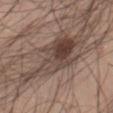Impression:
The lesion was tiled from a total-body skin photograph and was not biopsied.
Image and clinical context:
This image is a 15 mm lesion crop taken from a total-body photograph. The tile uses white-light illumination. Automated image analysis of the tile measured a lesion color around L≈43 a*≈14 b*≈22 in CIELAB and a normalized border contrast of about 9. Longest diameter approximately 9 mm. A male patient in their mid- to late 50s. On the right thigh.